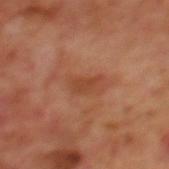follow-up=catalogued during a skin exam; not biopsied
subject=male, approximately 70 years of age
location=the mid back
imaging modality=~15 mm tile from a whole-body skin photo